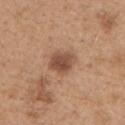Clinical impression:
The lesion was photographed on a routine skin check and not biopsied; there is no pathology result.
Background:
A 15 mm close-up tile from a total-body photography series done for melanoma screening. The patient is a male in their mid- to late 60s. Located on the chest.